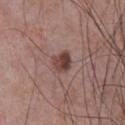The lesion was tiled from a total-body skin photograph and was not biopsied. Captured under white-light illumination. A male patient about 65 years old. Approximately 2.5 mm at its widest. From the chest. A region of skin cropped from a whole-body photographic capture, roughly 15 mm wide.